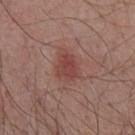Impression: The lesion was tiled from a total-body skin photograph and was not biopsied. Context: A 15 mm close-up extracted from a 3D total-body photography capture. A male subject, in their mid- to late 50s. This is a white-light tile. On the front of the torso. The lesion-visualizer software estimated a border-irregularity index near 3/10, a within-lesion color-variation index near 3/10, and a peripheral color-asymmetry measure near 1. And it measured a nevus-likeness score of about 70/100 and a detector confidence of about 100 out of 100 that the crop contains a lesion. Approximately 3.5 mm at its widest.